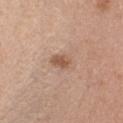Recorded during total-body skin imaging; not selected for excision or biopsy. The tile uses white-light illumination. The subject is a female roughly 30 years of age. A region of skin cropped from a whole-body photographic capture, roughly 15 mm wide. Approximately 2.5 mm at its widest. Automated image analysis of the tile measured an area of roughly 4 mm² and an eccentricity of roughly 0.75. It also reported a lesion color around L≈55 a*≈20 b*≈30 in CIELAB and a normalized lesion–skin contrast near 7.5. And it measured border irregularity of about 2 on a 0–10 scale, internal color variation of about 1.5 on a 0–10 scale, and peripheral color asymmetry of about 0.5. From the chest.